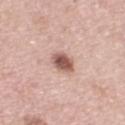Part of a total-body skin-imaging series; this lesion was reviewed on a skin check and was not flagged for biopsy.
This is a white-light tile.
A female patient, roughly 50 years of age.
A roughly 15 mm field-of-view crop from a total-body skin photograph.
Approximately 2.5 mm at its widest.
From the left upper arm.